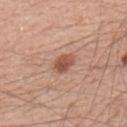biopsy_status: not biopsied; imaged during a skin examination
image:
  source: total-body photography crop
  field_of_view_mm: 15
automated_metrics:
  area_mm2_approx: 5.0
  eccentricity: 0.75
  shape_asymmetry: 0.2
  border_irregularity_0_10: 2.0
  color_variation_0_10: 3.0
  peripheral_color_asymmetry: 1.0
site: upper back
lighting: white-light
patient:
  sex: male
  age_approx: 55